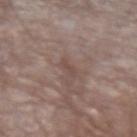Q: Is there a histopathology result?
A: no biopsy performed (imaged during a skin exam)
Q: Illumination type?
A: white-light illumination
Q: Automated lesion metrics?
A: an area of roughly 2 mm², a shape eccentricity near 0.95, and a shape-asymmetry score of about 0.4 (0 = symmetric); a mean CIELAB color near L≈47 a*≈17 b*≈21, about 6 CIELAB-L* units darker than the surrounding skin, and a normalized lesion–skin contrast near 5; a border-irregularity rating of about 5/10 and a within-lesion color-variation index near 0/10
Q: Lesion location?
A: the left upper arm
Q: How was this image acquired?
A: ~15 mm tile from a whole-body skin photo
Q: Patient demographics?
A: male, aged 63–67
Q: How large is the lesion?
A: ~3 mm (longest diameter)